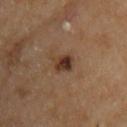| key | value |
|---|---|
| workup | imaged on a skin check; not biopsied |
| image source | total-body-photography crop, ~15 mm field of view |
| site | the chest |
| tile lighting | cross-polarized |
| subject | male, in their mid- to late 60s |
| lesion size | ~3 mm (longest diameter) |